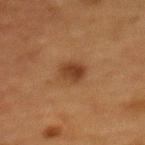{
  "lesion_size": {
    "long_diameter_mm_approx": 2.5
  },
  "patient": {
    "sex": "female",
    "age_approx": 50
  },
  "image": {
    "source": "total-body photography crop",
    "field_of_view_mm": 15
  },
  "site": "mid back"
}